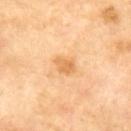Assessment:
This lesion was catalogued during total-body skin photography and was not selected for biopsy.
Background:
Cropped from a whole-body photographic skin survey; the tile spans about 15 mm. The lesion is on the mid back. The patient is a male aged 68 to 72.